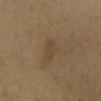Part of a total-body skin-imaging series; this lesion was reviewed on a skin check and was not flagged for biopsy.